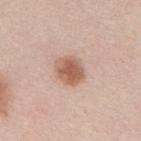notes=no biopsy performed (imaged during a skin exam) | diameter=about 3.5 mm | location=the mid back | image source=15 mm crop, total-body photography | subject=male, roughly 45 years of age | automated lesion analysis=a footprint of about 8 mm² and an eccentricity of roughly 0.6; a border-irregularity index near 1.5/10; a nevus-likeness score of about 100/100 and a lesion-detection confidence of about 100/100.